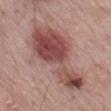workup = imaged on a skin check; not biopsied
site = the mid back
image source = 15 mm crop, total-body photography
patient = male, in their 70s